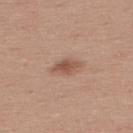{"biopsy_status": "not biopsied; imaged during a skin examination", "patient": {"sex": "male", "age_approx": 35}, "lesion_size": {"long_diameter_mm_approx": 3.5}, "image": {"source": "total-body photography crop", "field_of_view_mm": 15}, "site": "upper back"}